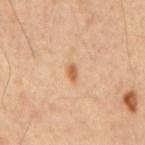Findings:
* notes — total-body-photography surveillance lesion; no biopsy
* subject — male, aged 58 to 62
* body site — the back
* image — 15 mm crop, total-body photography
* tile lighting — cross-polarized
* size — ≈2 mm